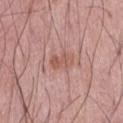Impression: This lesion was catalogued during total-body skin photography and was not selected for biopsy. Background: The tile uses white-light illumination. Approximately 3 mm at its widest. The patient is a male roughly 55 years of age. From the abdomen. Automated image analysis of the tile measured a lesion–skin lightness drop of about 7 and a normalized border contrast of about 6. The analysis additionally found a border-irregularity index near 2.5/10, a color-variation rating of about 3/10, and peripheral color asymmetry of about 1. The analysis additionally found a nevus-likeness score of about 0/100 and a lesion-detection confidence of about 100/100. A lesion tile, about 15 mm wide, cut from a 3D total-body photograph.